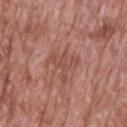biopsy_status: not biopsied; imaged during a skin examination
automated_metrics:
  border_irregularity_0_10: 8.5
  color_variation_0_10: 2.0
  peripheral_color_asymmetry: 1.0
  nevus_likeness_0_100: 0
  lesion_detection_confidence_0_100: 100
image:
  source: total-body photography crop
  field_of_view_mm: 15
site: upper back
patient:
  sex: male
  age_approx: 70
lesion_size:
  long_diameter_mm_approx: 4.0